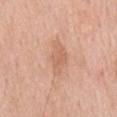<lesion>
  <biopsy_status>not biopsied; imaged during a skin examination</biopsy_status>
  <automated_metrics>
    <border_irregularity_0_10>2.5</border_irregularity_0_10>
    <color_variation_0_10>2.0</color_variation_0_10>
    <peripheral_color_asymmetry>0.5</peripheral_color_asymmetry>
  </automated_metrics>
  <lesion_size>
    <long_diameter_mm_approx>4.5</long_diameter_mm_approx>
  </lesion_size>
  <image>
    <source>total-body photography crop</source>
    <field_of_view_mm>15</field_of_view_mm>
  </image>
  <site>back</site>
  <lighting>white-light</lighting>
  <patient>
    <sex>male</sex>
    <age_approx>60</age_approx>
  </patient>
</lesion>